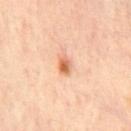| field | value |
|---|---|
| workup | no biopsy performed (imaged during a skin exam) |
| subject | male, aged approximately 65 |
| anatomic site | the chest |
| image | total-body-photography crop, ~15 mm field of view |
| lesion size | ≈2.5 mm |